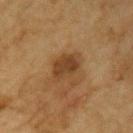Recorded during total-body skin imaging; not selected for excision or biopsy. A lesion tile, about 15 mm wide, cut from a 3D total-body photograph. A male patient, about 85 years old or older. Imaged with cross-polarized lighting. About 3.5 mm across. From the upper back.